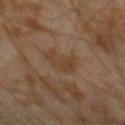biopsy_status: not biopsied; imaged during a skin examination
image:
  source: total-body photography crop
  field_of_view_mm: 15
site: left forearm
patient:
  sex: male
  age_approx: 45